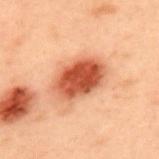workup: no biopsy performed (imaged during a skin exam); imaging modality: ~15 mm crop, total-body skin-cancer survey; body site: the upper back; lighting: cross-polarized; lesion size: ≈6 mm; patient: male, in their mid- to late 50s.